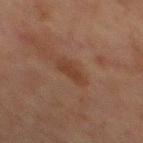<case>
<biopsy_status>not biopsied; imaged during a skin examination</biopsy_status>
<site>front of the torso</site>
<image>
  <source>total-body photography crop</source>
  <field_of_view_mm>15</field_of_view_mm>
</image>
<patient>
  <sex>male</sex>
  <age_approx>65</age_approx>
</patient>
</case>